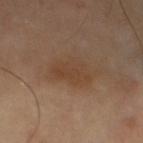biopsy status: total-body-photography surveillance lesion; no biopsy | subject: male, aged around 65 | acquisition: ~15 mm tile from a whole-body skin photo | location: the left thigh | tile lighting: cross-polarized.